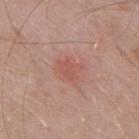workup: no biopsy performed (imaged during a skin exam); body site: the mid back; image source: ~15 mm tile from a whole-body skin photo; tile lighting: white-light illumination; subject: male, about 55 years old; diameter: ~3 mm (longest diameter).